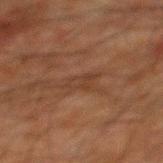Recorded during total-body skin imaging; not selected for excision or biopsy.
A male patient about 60 years old.
Cropped from a total-body skin-imaging series; the visible field is about 15 mm.
Imaged with cross-polarized lighting.
About 3 mm across.
An algorithmic analysis of the crop reported a footprint of about 3.5 mm², an eccentricity of roughly 0.8, and a shape-asymmetry score of about 0.3 (0 = symmetric). It also reported a within-lesion color-variation index near 1.5/10 and a peripheral color-asymmetry measure near 0.5. The software also gave an automated nevus-likeness rating near 0 out of 100.
The lesion is on the mid back.